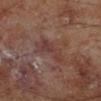<lesion>
<image>
  <source>total-body photography crop</source>
  <field_of_view_mm>15</field_of_view_mm>
</image>
<site>left lower leg</site>
<patient>
  <sex>male</sex>
  <age_approx>70</age_approx>
</patient>
</lesion>